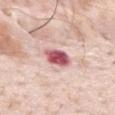The lesion was photographed on a routine skin check and not biopsied; there is no pathology result. A 15 mm close-up extracted from a 3D total-body photography capture. From the back. A male subject, in their mid-30s. The tile uses white-light illumination. About 3 mm across. Automated tile analysis of the lesion measured a footprint of about 6.5 mm², an outline eccentricity of about 0.5 (0 = round, 1 = elongated), and a symmetry-axis asymmetry near 0.15. And it measured a lesion color around L≈57 a*≈32 b*≈20 in CIELAB, about 20 CIELAB-L* units darker than the surrounding skin, and a lesion-to-skin contrast of about 12 (normalized; higher = more distinct). And it measured internal color variation of about 5.5 on a 0–10 scale. The analysis additionally found a nevus-likeness score of about 5/100 and lesion-presence confidence of about 100/100.